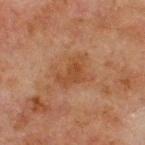| key | value |
|---|---|
| workup | total-body-photography surveillance lesion; no biopsy |
| patient | male, about 65 years old |
| automated lesion analysis | a footprint of about 10 mm², an eccentricity of roughly 0.65, and a shape-asymmetry score of about 0.35 (0 = symmetric); an average lesion color of about L≈38 a*≈19 b*≈30 (CIELAB), roughly 6 lightness units darker than nearby skin, and a normalized border contrast of about 6; a border-irregularity rating of about 5/10 and internal color variation of about 2.5 on a 0–10 scale |
| diameter | about 4 mm |
| acquisition | 15 mm crop, total-body photography |
| location | the chest |
| illumination | cross-polarized illumination |